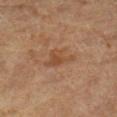workup: total-body-photography surveillance lesion; no biopsy
anatomic site: the leg
subject: female, in their 70s
TBP lesion metrics: a footprint of about 5.5 mm² and an eccentricity of roughly 0.7; a color-variation rating of about 2.5/10; lesion-presence confidence of about 100/100
image: ~15 mm crop, total-body skin-cancer survey
lighting: cross-polarized illumination
lesion size: ≈3.5 mm